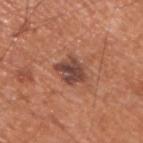The lesion was photographed on a routine skin check and not biopsied; there is no pathology result.
A 15 mm close-up extracted from a 3D total-body photography capture.
On the back.
The lesion's longest dimension is about 4 mm.
The patient is a male aged around 55.
This is a white-light tile.